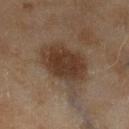Impression: Imaged during a routine full-body skin examination; the lesion was not biopsied and no histopathology is available. Context: A female patient, in their 60s. This image is a 15 mm lesion crop taken from a total-body photograph. The lesion is located on the left lower leg.